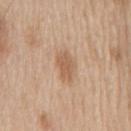No biopsy was performed on this lesion — it was imaged during a full skin examination and was not determined to be concerning. A lesion tile, about 15 mm wide, cut from a 3D total-body photograph. From the back. The subject is a male approximately 60 years of age.